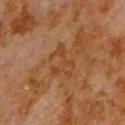The lesion was photographed on a routine skin check and not biopsied; there is no pathology result. This image is a 15 mm lesion crop taken from a total-body photograph. A male subject in their 80s. The lesion is located on the upper back.